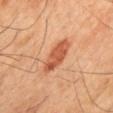{
  "lesion_size": {
    "long_diameter_mm_approx": 5.0
  },
  "lighting": "cross-polarized",
  "patient": {
    "sex": "male",
    "age_approx": 45
  },
  "image": {
    "source": "total-body photography crop",
    "field_of_view_mm": 15
  },
  "site": "mid back",
  "automated_metrics": {
    "vs_skin_darker_L": 12.0,
    "border_irregularity_0_10": 2.5,
    "color_variation_0_10": 4.0,
    "peripheral_color_asymmetry": 1.5
  }
}